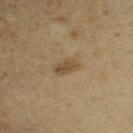Impression: This lesion was catalogued during total-body skin photography and was not selected for biopsy. Context: The lesion is located on the right upper arm. The patient is a male aged approximately 60. A region of skin cropped from a whole-body photographic capture, roughly 15 mm wide.